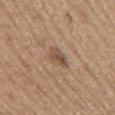The lesion was photographed on a routine skin check and not biopsied; there is no pathology result. The tile uses white-light illumination. This image is a 15 mm lesion crop taken from a total-body photograph. A male subject aged approximately 65. From the right upper arm.